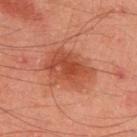No biopsy was performed on this lesion — it was imaged during a full skin examination and was not determined to be concerning.